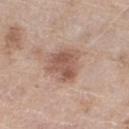<lesion>
  <biopsy_status>not biopsied; imaged during a skin examination</biopsy_status>
  <patient>
    <sex>female</sex>
    <age_approx>75</age_approx>
  </patient>
  <lesion_size>
    <long_diameter_mm_approx>4.0</long_diameter_mm_approx>
  </lesion_size>
  <lighting>white-light</lighting>
  <image>
    <source>total-body photography crop</source>
    <field_of_view_mm>15</field_of_view_mm>
  </image>
  <site>right thigh</site>
</lesion>